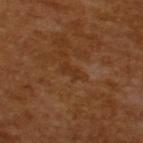biopsy_status: not biopsied; imaged during a skin examination
image:
  source: total-body photography crop
  field_of_view_mm: 15
lesion_size:
  long_diameter_mm_approx: 2.5
patient:
  sex: male
  age_approx: 65
automated_metrics:
  eccentricity: 0.9
  shape_asymmetry: 0.4
  cielab_L: 31
  cielab_a: 21
  cielab_b: 32
  vs_skin_contrast_norm: 5.0
  border_irregularity_0_10: 4.5
  color_variation_0_10: 0.0
lighting: cross-polarized